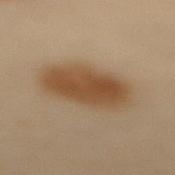notes: catalogued during a skin exam; not biopsied | patient: female, approximately 60 years of age | anatomic site: the upper back | image source: ~15 mm tile from a whole-body skin photo | size: ≈7 mm | tile lighting: cross-polarized illumination.